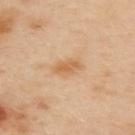The lesion was tiled from a total-body skin photograph and was not biopsied.
The lesion is on the upper back.
Measured at roughly 3.5 mm in maximum diameter.
The subject is a female about 40 years old.
Captured under cross-polarized illumination.
A region of skin cropped from a whole-body photographic capture, roughly 15 mm wide.